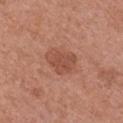Part of a total-body skin-imaging series; this lesion was reviewed on a skin check and was not flagged for biopsy. The lesion is on the chest. Captured under white-light illumination. A region of skin cropped from a whole-body photographic capture, roughly 15 mm wide. A female patient, aged 48–52. Longest diameter approximately 4 mm. Automated image analysis of the tile measured a footprint of about 9.5 mm², an eccentricity of roughly 0.7, and a shape-asymmetry score of about 0.25 (0 = symmetric). It also reported a mean CIELAB color near L≈50 a*≈24 b*≈30, a lesion–skin lightness drop of about 8, and a lesion-to-skin contrast of about 6 (normalized; higher = more distinct). And it measured a border-irregularity rating of about 2.5/10, internal color variation of about 2.5 on a 0–10 scale, and peripheral color asymmetry of about 1.